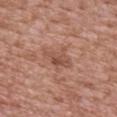Captured during whole-body skin photography for melanoma surveillance; the lesion was not biopsied. Approximately 3.5 mm at its widest. A male subject aged 43 to 47. This is a white-light tile. From the mid back. A 15 mm close-up tile from a total-body photography series done for melanoma screening.